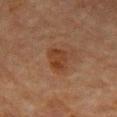Q: Was a biopsy performed?
A: no biopsy performed (imaged during a skin exam)
Q: What did automated image analysis measure?
A: border irregularity of about 3 on a 0–10 scale, internal color variation of about 2.5 on a 0–10 scale, and a peripheral color-asymmetry measure near 0.5
Q: How was this image acquired?
A: 15 mm crop, total-body photography
Q: Patient demographics?
A: male, about 80 years old
Q: What is the lesion's diameter?
A: ~3.5 mm (longest diameter)
Q: Lesion location?
A: the chest